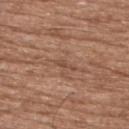Background: A male patient, aged 73–77. An algorithmic analysis of the crop reported a lesion area of about 1.5 mm², an outline eccentricity of about 0.95 (0 = round, 1 = elongated), and two-axis asymmetry of about 0.45. The software also gave an average lesion color of about L≈48 a*≈20 b*≈29 (CIELAB), about 7 CIELAB-L* units darker than the surrounding skin, and a normalized lesion–skin contrast near 5.5. The tile uses white-light illumination. The recorded lesion diameter is about 2.5 mm. From the upper back. A 15 mm crop from a total-body photograph taken for skin-cancer surveillance.